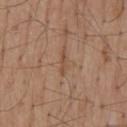workup: total-body-photography surveillance lesion; no biopsy | image-analysis metrics: an outline eccentricity of about 0.95 (0 = round, 1 = elongated) and two-axis asymmetry of about 0.4; a mean CIELAB color near L≈49 a*≈20 b*≈30 and roughly 7 lightness units darker than nearby skin; a border-irregularity index near 4/10 and radial color variation of about 0 | acquisition: 15 mm crop, total-body photography | patient: male, aged 53–57 | body site: the mid back.